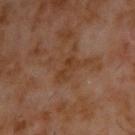This lesion was catalogued during total-body skin photography and was not selected for biopsy. The patient is a male aged 58 to 62. About 3 mm across. Located on the upper back. A 15 mm close-up tile from a total-body photography series done for melanoma screening. The tile uses cross-polarized illumination. The lesion-visualizer software estimated roughly 6 lightness units darker than nearby skin. It also reported a classifier nevus-likeness of about 0/100 and a detector confidence of about 100 out of 100 that the crop contains a lesion.